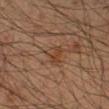A close-up tile cropped from a whole-body skin photograph, about 15 mm across. Captured under cross-polarized illumination. Located on the right forearm. Automated tile analysis of the lesion measured a footprint of about 4.5 mm², an outline eccentricity of about 0.5 (0 = round, 1 = elongated), and two-axis asymmetry of about 0.45. It also reported a nevus-likeness score of about 0/100 and lesion-presence confidence of about 100/100. A male subject approximately 35 years of age.